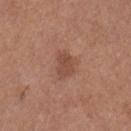Assessment: No biopsy was performed on this lesion — it was imaged during a full skin examination and was not determined to be concerning. Image and clinical context: The patient is a female in their mid- to late 50s. Captured under white-light illumination. From the leg. A 15 mm crop from a total-body photograph taken for skin-cancer surveillance. About 3 mm across.